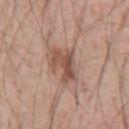Recorded during total-body skin imaging; not selected for excision or biopsy. The lesion is located on the chest. Imaged with white-light lighting. Longest diameter approximately 4 mm. A male patient in their mid-50s. Cropped from a whole-body photographic skin survey; the tile spans about 15 mm.